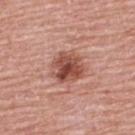This image is a 15 mm lesion crop taken from a total-body photograph.
On the upper back.
Measured at roughly 4 mm in maximum diameter.
A male patient, about 70 years old.